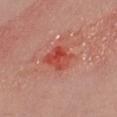No biopsy was performed on this lesion — it was imaged during a full skin examination and was not determined to be concerning.
A close-up tile cropped from a whole-body skin photograph, about 15 mm across.
Longest diameter approximately 3.5 mm.
The patient is a female in their 30s.
Automated tile analysis of the lesion measured an area of roughly 9 mm² and a shape-asymmetry score of about 0.25 (0 = symmetric). The software also gave border irregularity of about 3 on a 0–10 scale, a color-variation rating of about 8/10, and radial color variation of about 3.
The tile uses white-light illumination.
The lesion is on the leg.